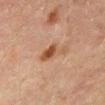Q: Was this lesion biopsied?
A: no biopsy performed (imaged during a skin exam)
Q: How was the tile lit?
A: cross-polarized
Q: How large is the lesion?
A: about 4 mm
Q: What are the patient's age and sex?
A: male, aged around 60
Q: Where on the body is the lesion?
A: the abdomen
Q: How was this image acquired?
A: total-body-photography crop, ~15 mm field of view